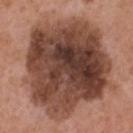biopsy_status: not biopsied; imaged during a skin examination
site: head or neck
image:
  source: total-body photography crop
  field_of_view_mm: 15
patient:
  sex: male
  age_approx: 50